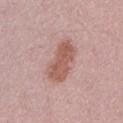The lesion was tiled from a total-body skin photograph and was not biopsied. The lesion's longest dimension is about 5.5 mm. A female patient, aged 43–47. This is a white-light tile. Automated image analysis of the tile measured a mean CIELAB color near L≈56 a*≈22 b*≈25 and a normalized lesion–skin contrast near 8. The software also gave a border-irregularity rating of about 2.5/10. The software also gave a classifier nevus-likeness of about 30/100 and a lesion-detection confidence of about 100/100. Located on the arm. A roughly 15 mm field-of-view crop from a total-body skin photograph.